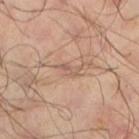The lesion was tiled from a total-body skin photograph and was not biopsied. The recorded lesion diameter is about 3 mm. On the left lower leg. This image is a 15 mm lesion crop taken from a total-body photograph. A male subject roughly 65 years of age. The tile uses cross-polarized illumination. Automated tile analysis of the lesion measured a mean CIELAB color near L≈53 a*≈17 b*≈26, a lesion–skin lightness drop of about 7, and a normalized lesion–skin contrast near 5. It also reported a border-irregularity rating of about 6.5/10 and a peripheral color-asymmetry measure near 0.